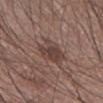Assessment:
Part of a total-body skin-imaging series; this lesion was reviewed on a skin check and was not flagged for biopsy.
Image and clinical context:
Captured under white-light illumination. A male subject approximately 55 years of age. The lesion is located on the right forearm. Cropped from a whole-body photographic skin survey; the tile spans about 15 mm. Approximately 4 mm at its widest.